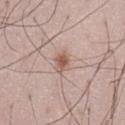{
  "biopsy_status": "not biopsied; imaged during a skin examination",
  "site": "left thigh",
  "image": {
    "source": "total-body photography crop",
    "field_of_view_mm": 15
  },
  "patient": {
    "sex": "male",
    "age_approx": 50
  },
  "automated_metrics": {
    "cielab_L": 57,
    "cielab_a": 18,
    "cielab_b": 26,
    "vs_skin_darker_L": 11.0,
    "border_irregularity_0_10": 2.5,
    "color_variation_0_10": 2.5,
    "peripheral_color_asymmetry": 1.0,
    "nevus_likeness_0_100": 85,
    "lesion_detection_confidence_0_100": 100
  },
  "lesion_size": {
    "long_diameter_mm_approx": 3.0
  }
}